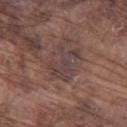Findings:
– workup: total-body-photography surveillance lesion; no biopsy
– subject: male, in their mid-70s
– acquisition: total-body-photography crop, ~15 mm field of view
– location: the leg
– lesion size: ~4.5 mm (longest diameter)